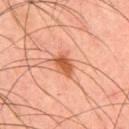Part of a total-body skin-imaging series; this lesion was reviewed on a skin check and was not flagged for biopsy.
On the back.
This image is a 15 mm lesion crop taken from a total-body photograph.
The total-body-photography lesion software estimated a lesion area of about 5.5 mm², a shape eccentricity near 0.75, and a symmetry-axis asymmetry near 0.2. The software also gave a classifier nevus-likeness of about 95/100 and a lesion-detection confidence of about 100/100.
Imaged with cross-polarized lighting.
Longest diameter approximately 3 mm.
A male patient, approximately 45 years of age.